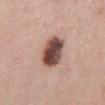biopsy status: no biopsy performed (imaged during a skin exam)
image: 15 mm crop, total-body photography
patient: male, approximately 70 years of age
location: the front of the torso